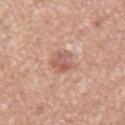Q: Is there a histopathology result?
A: no biopsy performed (imaged during a skin exam)
Q: Who is the patient?
A: male, roughly 75 years of age
Q: How large is the lesion?
A: ~3.5 mm (longest diameter)
Q: How was this image acquired?
A: ~15 mm tile from a whole-body skin photo
Q: Automated lesion metrics?
A: a footprint of about 7 mm² and an outline eccentricity of about 0.7 (0 = round, 1 = elongated); a mean CIELAB color near L≈59 a*≈23 b*≈27, roughly 9 lightness units darker than nearby skin, and a normalized border contrast of about 6; an automated nevus-likeness rating near 0 out of 100 and a lesion-detection confidence of about 100/100
Q: Where on the body is the lesion?
A: the back
Q: How was the tile lit?
A: white-light